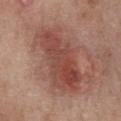biopsy_status: not biopsied; imaged during a skin examination
lesion_size:
  long_diameter_mm_approx: 8.5
site: chest
automated_metrics:
  cielab_L: 47
  cielab_a: 24
  cielab_b: 26
  vs_skin_darker_L: 10.0
  vs_skin_contrast_norm: 8.0
  nevus_likeness_0_100: 0
  lesion_detection_confidence_0_100: 100
patient:
  sex: female
  age_approx: 50
lighting: white-light
image:
  source: total-body photography crop
  field_of_view_mm: 15